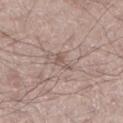Assessment:
Captured during whole-body skin photography for melanoma surveillance; the lesion was not biopsied.
Background:
From the left lower leg. A male subject, about 35 years old. Cropped from a total-body skin-imaging series; the visible field is about 15 mm.